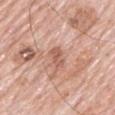Clinical impression:
No biopsy was performed on this lesion — it was imaged during a full skin examination and was not determined to be concerning.
Context:
A 15 mm close-up extracted from a 3D total-body photography capture. The lesion's longest dimension is about 3 mm. Captured under white-light illumination. The lesion is located on the mid back. A male patient, approximately 80 years of age.